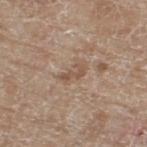Impression: Part of a total-body skin-imaging series; this lesion was reviewed on a skin check and was not flagged for biopsy. Context: Imaged with white-light lighting. The lesion is located on the right thigh. The total-body-photography lesion software estimated an automated nevus-likeness rating near 0 out of 100 and a lesion-detection confidence of about 95/100. The recorded lesion diameter is about 2.5 mm. The patient is a female roughly 75 years of age. A roughly 15 mm field-of-view crop from a total-body skin photograph.